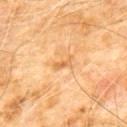No biopsy was performed on this lesion — it was imaged during a full skin examination and was not determined to be concerning.
A 15 mm crop from a total-body photograph taken for skin-cancer surveillance.
Measured at roughly 2.5 mm in maximum diameter.
A male subject aged 58–62.
The lesion is located on the chest.
This is a cross-polarized tile.
The lesion-visualizer software estimated a footprint of about 2 mm², an outline eccentricity of about 0.9 (0 = round, 1 = elongated), and two-axis asymmetry of about 0.45. And it measured a lesion color around L≈65 a*≈23 b*≈45 in CIELAB and a lesion-to-skin contrast of about 6 (normalized; higher = more distinct).